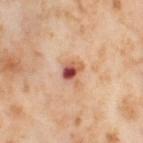Recorded during total-body skin imaging; not selected for excision or biopsy. A region of skin cropped from a whole-body photographic capture, roughly 15 mm wide. Measured at roughly 2.5 mm in maximum diameter. From the left thigh. The patient is a female roughly 55 years of age.